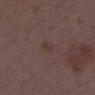The lesion was photographed on a routine skin check and not biopsied; there is no pathology result.
On the right thigh.
The patient is a female approximately 35 years of age.
Imaged with white-light lighting.
A 15 mm crop from a total-body photograph taken for skin-cancer surveillance.
Approximately 2.5 mm at its widest.